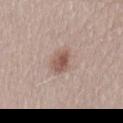Clinical impression:
The lesion was photographed on a routine skin check and not biopsied; there is no pathology result.
Clinical summary:
From the lower back. A female subject approximately 30 years of age. Automated image analysis of the tile measured internal color variation of about 4 on a 0–10 scale and radial color variation of about 1.5. And it measured a nevus-likeness score of about 90/100 and lesion-presence confidence of about 100/100. About 3 mm across. This is a white-light tile. A 15 mm close-up extracted from a 3D total-body photography capture.